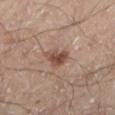{"automated_metrics": {"eccentricity": 0.8, "shape_asymmetry": 0.35, "nevus_likeness_0_100": 85, "lesion_detection_confidence_0_100": 100}, "lesion_size": {"long_diameter_mm_approx": 3.5}, "image": {"source": "total-body photography crop", "field_of_view_mm": 15}, "lighting": "cross-polarized", "site": "leg", "patient": {"sex": "male", "age_approx": 45}}